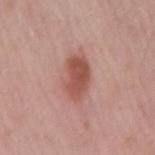biopsy status=imaged on a skin check; not biopsied | image source=15 mm crop, total-body photography | patient=male, aged 58–62 | automated metrics=a lesion color around L≈52 a*≈24 b*≈26 in CIELAB and a normalized border contrast of about 8.5; a nevus-likeness score of about 100/100 | size=≈5 mm | tile lighting=white-light illumination | body site=the back.